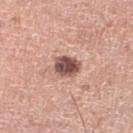The lesion was photographed on a routine skin check and not biopsied; there is no pathology result. The patient is a male about 60 years old. The lesion is located on the left lower leg. Imaged with white-light lighting. A 15 mm close-up extracted from a 3D total-body photography capture. The lesion's longest dimension is about 3.5 mm.